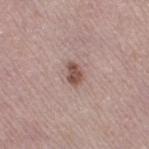The lesion is located on the right thigh.
A 15 mm crop from a total-body photograph taken for skin-cancer surveillance.
A female patient, about 50 years old.
This is a white-light tile.
The lesion's longest dimension is about 2.5 mm.